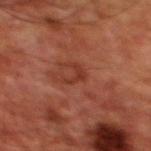No biopsy was performed on this lesion — it was imaged during a full skin examination and was not determined to be concerning.
Cropped from a whole-body photographic skin survey; the tile spans about 15 mm.
From the chest.
A male subject, in their 60s.
The total-body-photography lesion software estimated an area of roughly 2.5 mm² and a symmetry-axis asymmetry near 0.65. The software also gave a border-irregularity index near 7.5/10 and peripheral color asymmetry of about 0.
Measured at roughly 2.5 mm in maximum diameter.